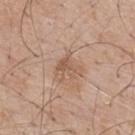The lesion was tiled from a total-body skin photograph and was not biopsied. About 3 mm across. Captured under white-light illumination. A male subject aged approximately 60. Cropped from a whole-body photographic skin survey; the tile spans about 15 mm. On the upper back.